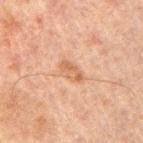biopsy_status: not biopsied; imaged during a skin examination
lesion_size:
  long_diameter_mm_approx: 3.0
automated_metrics:
  cielab_L: 47
  cielab_a: 19
  cielab_b: 28
  vs_skin_darker_L: 7.0
  border_irregularity_0_10: 2.5
  color_variation_0_10: 2.0
  peripheral_color_asymmetry: 0.5
lighting: cross-polarized
patient:
  sex: male
  age_approx: 60
site: left arm
image:
  source: total-body photography crop
  field_of_view_mm: 15